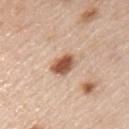| field | value |
|---|---|
| workup | total-body-photography surveillance lesion; no biopsy |
| imaging modality | ~15 mm crop, total-body skin-cancer survey |
| tile lighting | white-light illumination |
| diameter | ~3.5 mm (longest diameter) |
| automated metrics | a footprint of about 6.5 mm², an eccentricity of roughly 0.75, and a shape-asymmetry score of about 0.15 (0 = symmetric); a lesion color around L≈56 a*≈22 b*≈32 in CIELAB, a lesion–skin lightness drop of about 17, and a lesion-to-skin contrast of about 11 (normalized; higher = more distinct) |
| location | the chest |
| subject | male, approximately 45 years of age |